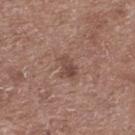notes=no biopsy performed (imaged during a skin exam); subject=male, aged 58–62; anatomic site=the upper back; lighting=white-light; image source=15 mm crop, total-body photography.